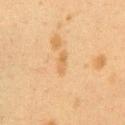<case>
  <biopsy_status>not biopsied; imaged during a skin examination</biopsy_status>
  <site>left upper arm</site>
  <image>
    <source>total-body photography crop</source>
    <field_of_view_mm>15</field_of_view_mm>
  </image>
  <lesion_size>
    <long_diameter_mm_approx>3.0</long_diameter_mm_approx>
  </lesion_size>
  <lighting>cross-polarized</lighting>
  <patient>
    <sex>female</sex>
    <age_approx>40</age_approx>
  </patient>
</case>